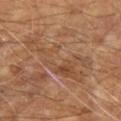<lesion>
  <biopsy_status>not biopsied; imaged during a skin examination</biopsy_status>
  <patient>
    <sex>male</sex>
    <age_approx>65</age_approx>
  </patient>
  <lighting>cross-polarized</lighting>
  <automated_metrics>
    <area_mm2_approx>23.0</area_mm2_approx>
    <shape_asymmetry>0.5</shape_asymmetry>
    <nevus_likeness_0_100>0</nevus_likeness_0_100>
    <lesion_detection_confidence_0_100>60</lesion_detection_confidence_0_100>
  </automated_metrics>
  <lesion_size>
    <long_diameter_mm_approx>8.5</long_diameter_mm_approx>
  </lesion_size>
  <image>
    <source>total-body photography crop</source>
    <field_of_view_mm>15</field_of_view_mm>
  </image>
  <site>right upper arm</site>
</lesion>